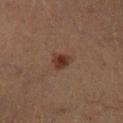The lesion was tiled from a total-body skin photograph and was not biopsied.